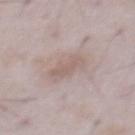Captured during whole-body skin photography for melanoma surveillance; the lesion was not biopsied. The lesion is located on the abdomen. A 15 mm close-up extracted from a 3D total-body photography capture. This is a white-light tile. A male subject, in their 50s.